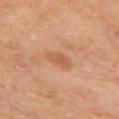Findings:
– notes · no biopsy performed (imaged during a skin exam)
– subject · male, approximately 70 years of age
– location · the arm
– illumination · cross-polarized illumination
– diameter · ~3 mm (longest diameter)
– acquisition · ~15 mm tile from a whole-body skin photo